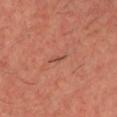The lesion was tiled from a total-body skin photograph and was not biopsied.
The lesion is located on the chest.
Imaged with cross-polarized lighting.
A lesion tile, about 15 mm wide, cut from a 3D total-body photograph.
A male subject, about 35 years old.